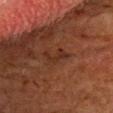<lesion>
  <biopsy_status>not biopsied; imaged during a skin examination</biopsy_status>
  <image>
    <source>total-body photography crop</source>
    <field_of_view_mm>15</field_of_view_mm>
  </image>
  <lighting>cross-polarized</lighting>
  <patient>
    <sex>male</sex>
    <age_approx>65</age_approx>
  </patient>
  <site>head or neck</site>
  <automated_metrics>
    <cielab_L>23</cielab_L>
    <cielab_a>19</cielab_a>
    <cielab_b>23</cielab_b>
    <vs_skin_darker_L>5.0</vs_skin_darker_L>
    <vs_skin_contrast_norm>6.0</vs_skin_contrast_norm>
    <border_irregularity_0_10>7.0</border_irregularity_0_10>
    <peripheral_color_asymmetry>0.0</peripheral_color_asymmetry>
    <lesion_detection_confidence_0_100>95</lesion_detection_confidence_0_100>
  </automated_metrics>
  <lesion_size>
    <long_diameter_mm_approx>3.0</long_diameter_mm_approx>
  </lesion_size>
</lesion>